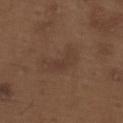Notes:
• biopsy status — total-body-photography surveillance lesion; no biopsy
• illumination — white-light
• patient — male, about 70 years old
• size — ≈5 mm
• image source — ~15 mm tile from a whole-body skin photo
• body site — the upper back